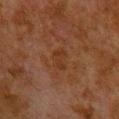follow-up: total-body-photography surveillance lesion; no biopsy
subject: male, aged approximately 80
lighting: cross-polarized illumination
acquisition: total-body-photography crop, ~15 mm field of view
site: the back
TBP lesion metrics: a border-irregularity index near 2.5/10, a color-variation rating of about 1/10, and a peripheral color-asymmetry measure near 0.5